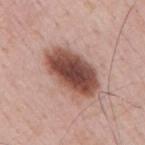{"biopsy_status": "not biopsied; imaged during a skin examination", "image": {"source": "total-body photography crop", "field_of_view_mm": 15}, "lighting": "white-light", "lesion_size": {"long_diameter_mm_approx": 7.0}, "site": "right upper arm", "patient": {"sex": "male", "age_approx": 50}}